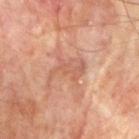Longest diameter approximately 4 mm. A lesion tile, about 15 mm wide, cut from a 3D total-body photograph. This is a cross-polarized tile. On the right upper arm. A male subject aged around 75.